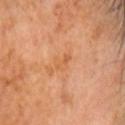{"biopsy_status": "not biopsied; imaged during a skin examination", "patient": {"sex": "male", "age_approx": 60}, "site": "head or neck", "image": {"source": "total-body photography crop", "field_of_view_mm": 15}, "lighting": "cross-polarized", "lesion_size": {"long_diameter_mm_approx": 2.5}}